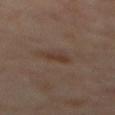Assessment: Imaged during a routine full-body skin examination; the lesion was not biopsied and no histopathology is available. Background: A roughly 15 mm field-of-view crop from a total-body skin photograph. The lesion is located on the mid back. Captured under cross-polarized illumination. The subject is a male aged 63 to 67. Measured at roughly 3 mm in maximum diameter. An algorithmic analysis of the crop reported a footprint of about 3 mm² and a shape eccentricity near 0.9.